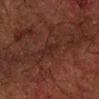Case summary:
• notes · no biopsy performed (imaged during a skin exam)
• image · 15 mm crop, total-body photography
• patient · male, aged 48 to 52
• site · the right forearm